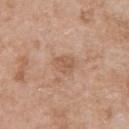<lesion>
  <biopsy_status>not biopsied; imaged during a skin examination</biopsy_status>
  <image>
    <source>total-body photography crop</source>
    <field_of_view_mm>15</field_of_view_mm>
  </image>
  <lesion_size>
    <long_diameter_mm_approx>2.5</long_diameter_mm_approx>
  </lesion_size>
  <patient>
    <sex>male</sex>
    <age_approx>50</age_approx>
  </patient>
  <lighting>white-light</lighting>
  <site>chest</site>
</lesion>